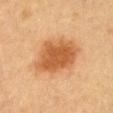This lesion was catalogued during total-body skin photography and was not selected for biopsy.
The subject is a female aged around 30.
Located on the chest.
The lesion-visualizer software estimated a lesion area of about 21 mm², a shape eccentricity near 0.65, and a shape-asymmetry score of about 0.2 (0 = symmetric). The software also gave border irregularity of about 2.5 on a 0–10 scale and radial color variation of about 1. It also reported an automated nevus-likeness rating near 100 out of 100.
A lesion tile, about 15 mm wide, cut from a 3D total-body photograph.